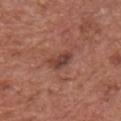Part of a total-body skin-imaging series; this lesion was reviewed on a skin check and was not flagged for biopsy.
The patient is a male roughly 75 years of age.
The total-body-photography lesion software estimated an area of roughly 4.5 mm² and a shape eccentricity near 0.8. It also reported a mean CIELAB color near L≈41 a*≈24 b*≈25, about 10 CIELAB-L* units darker than the surrounding skin, and a normalized lesion–skin contrast near 8. The software also gave a border-irregularity index near 3/10, a color-variation rating of about 4/10, and radial color variation of about 1.5. The analysis additionally found a nevus-likeness score of about 15/100 and a lesion-detection confidence of about 100/100.
The tile uses white-light illumination.
A 15 mm crop from a total-body photograph taken for skin-cancer surveillance.
The lesion is located on the chest.
Measured at roughly 3 mm in maximum diameter.